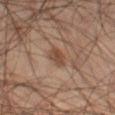The tile uses cross-polarized illumination. The lesion-visualizer software estimated an area of roughly 4 mm², an outline eccentricity of about 0.75 (0 = round, 1 = elongated), and two-axis asymmetry of about 0.2. The software also gave a lesion color around L≈42 a*≈17 b*≈26 in CIELAB and a lesion-to-skin contrast of about 7 (normalized; higher = more distinct). The analysis additionally found border irregularity of about 1.5 on a 0–10 scale, internal color variation of about 2.5 on a 0–10 scale, and radial color variation of about 1. Longest diameter approximately 3 mm. The lesion is located on the left thigh. A male patient, aged around 55. A close-up tile cropped from a whole-body skin photograph, about 15 mm across.